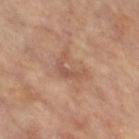biopsy_status: not biopsied; imaged during a skin examination
patient:
  sex: female
  age_approx: 65
automated_metrics:
  border_irregularity_0_10: 7.5
  color_variation_0_10: 1.5
  peripheral_color_asymmetry: 0.5
lighting: cross-polarized
image:
  source: total-body photography crop
  field_of_view_mm: 15
site: left leg
lesion_size:
  long_diameter_mm_approx: 4.0